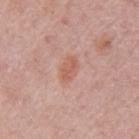Impression:
This lesion was catalogued during total-body skin photography and was not selected for biopsy.
Image and clinical context:
Longest diameter approximately 3 mm. This is a white-light tile. A female subject, about 65 years old. A 15 mm close-up tile from a total-body photography series done for melanoma screening. Located on the left upper arm. Automated tile analysis of the lesion measured internal color variation of about 2.5 on a 0–10 scale. The analysis additionally found a lesion-detection confidence of about 100/100.